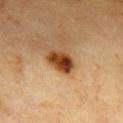Clinical impression:
This lesion was catalogued during total-body skin photography and was not selected for biopsy.
Image and clinical context:
A 15 mm close-up extracted from a 3D total-body photography capture. The total-body-photography lesion software estimated an average lesion color of about L≈33 a*≈21 b*≈33 (CIELAB), roughly 16 lightness units darker than nearby skin, and a normalized border contrast of about 14. A female subject in their mid-50s. The tile uses cross-polarized illumination. From the chest. The lesion's longest dimension is about 3.5 mm.